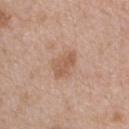The lesion was photographed on a routine skin check and not biopsied; there is no pathology result.
Located on the back.
A 15 mm close-up extracted from a 3D total-body photography capture.
Approximately 3.5 mm at its widest.
The subject is a female in their mid- to late 40s.